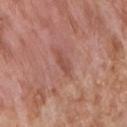Captured during whole-body skin photography for melanoma surveillance; the lesion was not biopsied. A roughly 15 mm field-of-view crop from a total-body skin photograph. Captured under white-light illumination. Located on the chest. Approximately 3 mm at its widest. The patient is a male about 65 years old. The total-body-photography lesion software estimated a lesion-to-skin contrast of about 6 (normalized; higher = more distinct). It also reported a border-irregularity index near 3.5/10, internal color variation of about 0 on a 0–10 scale, and peripheral color asymmetry of about 0.